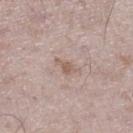No biopsy was performed on this lesion — it was imaged during a full skin examination and was not determined to be concerning.
A male patient, approximately 75 years of age.
Imaged with white-light lighting.
The lesion is located on the right lower leg.
About 2.5 mm across.
The total-body-photography lesion software estimated an area of roughly 2.5 mm² and an eccentricity of roughly 0.85. And it measured a mean CIELAB color near L≈58 a*≈16 b*≈25 and a lesion–skin lightness drop of about 8.
A lesion tile, about 15 mm wide, cut from a 3D total-body photograph.